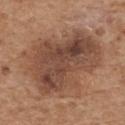The lesion was photographed on a routine skin check and not biopsied; there is no pathology result.
Located on the back.
The patient is a male roughly 70 years of age.
A roughly 15 mm field-of-view crop from a total-body skin photograph.